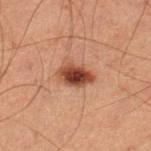{"biopsy_status": "not biopsied; imaged during a skin examination", "site": "left lower leg", "lighting": "cross-polarized", "patient": {"sex": "male", "age_approx": 60}, "lesion_size": {"long_diameter_mm_approx": 4.0}, "image": {"source": "total-body photography crop", "field_of_view_mm": 15}}